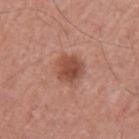Part of a total-body skin-imaging series; this lesion was reviewed on a skin check and was not flagged for biopsy. This is a white-light tile. A male patient in their mid-40s. A close-up tile cropped from a whole-body skin photograph, about 15 mm across. The lesion is located on the arm. An algorithmic analysis of the crop reported a mean CIELAB color near L≈49 a*≈25 b*≈29 and a normalized border contrast of about 8.